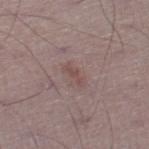{"lighting": "white-light", "site": "right lower leg", "lesion_size": {"long_diameter_mm_approx": 3.0}, "image": {"source": "total-body photography crop", "field_of_view_mm": 15}, "patient": {"sex": "male", "age_approx": 50}}